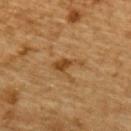Imaged during a routine full-body skin examination; the lesion was not biopsied and no histopathology is available.
The lesion is located on the upper back.
This image is a 15 mm lesion crop taken from a total-body photograph.
A male subject approximately 85 years of age or older.